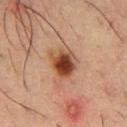Clinical impression:
No biopsy was performed on this lesion — it was imaged during a full skin examination and was not determined to be concerning.
Context:
Captured under cross-polarized illumination. Cropped from a total-body skin-imaging series; the visible field is about 15 mm. The lesion is located on the front of the torso. A male subject approximately 35 years of age.